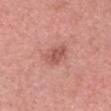imaging modality = ~15 mm crop, total-body skin-cancer survey; location = the head or neck; subject = female, roughly 40 years of age; lesion size = about 3.5 mm; tile lighting = white-light.